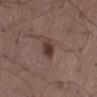Assessment: The lesion was tiled from a total-body skin photograph and was not biopsied. Image and clinical context: Cropped from a whole-body photographic skin survey; the tile spans about 15 mm. Located on the lower back. A male subject, aged 48–52. The tile uses white-light illumination. Automated image analysis of the tile measured a mean CIELAB color near L≈37 a*≈17 b*≈20, a lesion–skin lightness drop of about 11, and a lesion-to-skin contrast of about 9.5 (normalized; higher = more distinct).